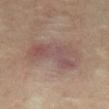{
  "biopsy_status": "not biopsied; imaged during a skin examination",
  "image": {
    "source": "total-body photography crop",
    "field_of_view_mm": 15
  },
  "site": "mid back",
  "lighting": "cross-polarized",
  "automated_metrics": {
    "area_mm2_approx": 18.0,
    "eccentricity": 0.9,
    "shape_asymmetry": 0.35
  },
  "lesion_size": {
    "long_diameter_mm_approx": 7.0
  },
  "patient": {
    "sex": "male",
    "age_approx": 35
  }
}